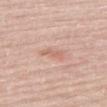follow-up = total-body-photography surveillance lesion; no biopsy | patient = male, approximately 70 years of age | automated metrics = a border-irregularity index near 4/10; lesion-presence confidence of about 100/100 | location = the right thigh | imaging modality = ~15 mm crop, total-body skin-cancer survey | illumination = white-light | size = ~2.5 mm (longest diameter).